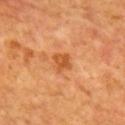This lesion was catalogued during total-body skin photography and was not selected for biopsy. A close-up tile cropped from a whole-body skin photograph, about 15 mm across. Captured under cross-polarized illumination. A male subject aged approximately 65. Longest diameter approximately 2.5 mm. The total-body-photography lesion software estimated a mean CIELAB color near L≈56 a*≈30 b*≈46 and a normalized lesion–skin contrast near 7. The analysis additionally found a nevus-likeness score of about 5/100 and a lesion-detection confidence of about 100/100. The lesion is located on the upper back.